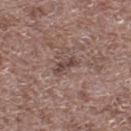{"biopsy_status": "not biopsied; imaged during a skin examination", "lesion_size": {"long_diameter_mm_approx": 3.0}, "image": {"source": "total-body photography crop", "field_of_view_mm": 15}, "lighting": "white-light", "patient": {"sex": "male", "age_approx": 70}, "site": "left lower leg"}